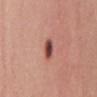biopsy status = no biopsy performed (imaged during a skin exam)
body site = the mid back
size = ≈2.5 mm
image = ~15 mm tile from a whole-body skin photo
illumination = white-light
TBP lesion metrics = a lesion color around L≈47 a*≈25 b*≈25 in CIELAB, roughly 16 lightness units darker than nearby skin, and a normalized lesion–skin contrast near 11; a border-irregularity index near 1/10 and radial color variation of about 2
patient = female, roughly 35 years of age